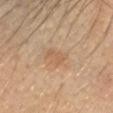No biopsy was performed on this lesion — it was imaged during a full skin examination and was not determined to be concerning.
The lesion's longest dimension is about 3 mm.
The tile uses cross-polarized illumination.
The subject is a female approximately 40 years of age.
Located on the head or neck.
Cropped from a total-body skin-imaging series; the visible field is about 15 mm.